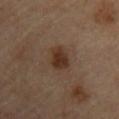Q: Who is the patient?
A: male, aged 83 or over
Q: What lighting was used for the tile?
A: cross-polarized illumination
Q: How large is the lesion?
A: ≈3 mm
Q: Automated lesion metrics?
A: a lesion area of about 5.5 mm², a shape eccentricity near 0.5, and a shape-asymmetry score of about 0.25 (0 = symmetric); an automated nevus-likeness rating near 95 out of 100 and a lesion-detection confidence of about 100/100
Q: How was this image acquired?
A: 15 mm crop, total-body photography
Q: What is the anatomic site?
A: the upper back